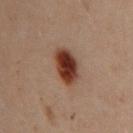Recorded during total-body skin imaging; not selected for excision or biopsy.
On the left arm.
A female patient, aged 28 to 32.
A roughly 15 mm field-of-view crop from a total-body skin photograph.